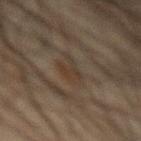biopsy_status: not biopsied; imaged during a skin examination
lesion_size:
  long_diameter_mm_approx: 3.5
lighting: cross-polarized
patient:
  sex: male
  age_approx: 65
image:
  source: total-body photography crop
  field_of_view_mm: 15
site: abdomen